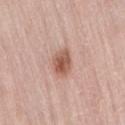Captured under white-light illumination. A 15 mm close-up tile from a total-body photography series done for melanoma screening. The subject is a female aged approximately 65. The lesion is located on the back.